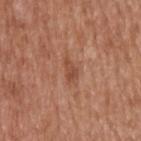Impression: Captured during whole-body skin photography for melanoma surveillance; the lesion was not biopsied. Clinical summary: The patient is a male approximately 65 years of age. This is a white-light tile. The lesion is on the upper back. A 15 mm close-up tile from a total-body photography series done for melanoma screening. About 3 mm across.